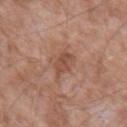Assessment:
Part of a total-body skin-imaging series; this lesion was reviewed on a skin check and was not flagged for biopsy.
Image and clinical context:
A male subject roughly 75 years of age. A 15 mm close-up extracted from a 3D total-body photography capture. The lesion-visualizer software estimated a footprint of about 5 mm² and a symmetry-axis asymmetry near 0.35. The software also gave a border-irregularity rating of about 3.5/10, a within-lesion color-variation index near 2.5/10, and a peripheral color-asymmetry measure near 1. The lesion is located on the left upper arm.